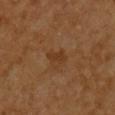biopsy_status: not biopsied; imaged during a skin examination
lighting: cross-polarized
lesion_size:
  long_diameter_mm_approx: 3.0
patient:
  sex: male
  age_approx: 60
image:
  source: total-body photography crop
  field_of_view_mm: 15
site: chest